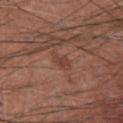biopsy status = total-body-photography surveillance lesion; no biopsy | size = about 3 mm | body site = the chest | automated metrics = a color-variation rating of about 1/10; a lesion-detection confidence of about 100/100 | imaging modality = total-body-photography crop, ~15 mm field of view | lighting = white-light | subject = male, about 60 years old.